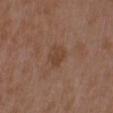Acquisition and patient details:
Captured under white-light illumination. The lesion is on the upper back. A 15 mm close-up tile from a total-body photography series done for melanoma screening. The lesion-visualizer software estimated about 7 CIELAB-L* units darker than the surrounding skin. The analysis additionally found a border-irregularity rating of about 3.5/10, a within-lesion color-variation index near 1.5/10, and peripheral color asymmetry of about 0.5. The patient is a female aged around 30. About 2.5 mm across.